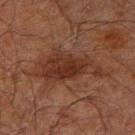Clinical impression: No biopsy was performed on this lesion — it was imaged during a full skin examination and was not determined to be concerning. Context: Captured under cross-polarized illumination. A male subject, aged 68–72. The recorded lesion diameter is about 7.5 mm. On the arm. A 15 mm crop from a total-body photograph taken for skin-cancer surveillance.